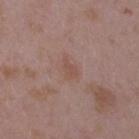{
  "biopsy_status": "not biopsied; imaged during a skin examination",
  "image": {
    "source": "total-body photography crop",
    "field_of_view_mm": 15
  },
  "patient": {
    "sex": "female",
    "age_approx": 35
  },
  "site": "right upper arm",
  "lighting": "white-light",
  "lesion_size": {
    "long_diameter_mm_approx": 2.5
  }
}